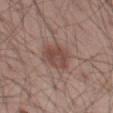notes: imaged on a skin check; not biopsied | tile lighting: white-light | image: total-body-photography crop, ~15 mm field of view | lesion diameter: ~4 mm (longest diameter) | image-analysis metrics: a footprint of about 10 mm², a shape eccentricity near 0.65, and two-axis asymmetry of about 0.15 | patient: male, aged around 55 | site: the left thigh.